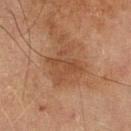Findings:
* biopsy status — no biopsy performed (imaged during a skin exam)
* location — the left lower leg
* image source — total-body-photography crop, ~15 mm field of view
* illumination — cross-polarized
* patient — male, aged around 70
* size — about 4.5 mm